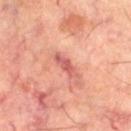The lesion was photographed on a routine skin check and not biopsied; there is no pathology result. A male subject, aged around 60. A close-up tile cropped from a whole-body skin photograph, about 15 mm across. Measured at roughly 3.5 mm in maximum diameter. The tile uses cross-polarized illumination. The lesion is on the right thigh.